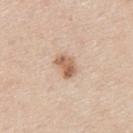This lesion was catalogued during total-body skin photography and was not selected for biopsy. Located on the upper back. A male subject in their mid-30s. The total-body-photography lesion software estimated a lesion area of about 5.5 mm² and a symmetry-axis asymmetry near 0.25. The analysis additionally found an average lesion color of about L≈62 a*≈20 b*≈31 (CIELAB), a lesion–skin lightness drop of about 13, and a normalized border contrast of about 8.5. A 15 mm crop from a total-body photograph taken for skin-cancer surveillance.